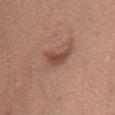This lesion was catalogued during total-body skin photography and was not selected for biopsy.
On the upper back.
The recorded lesion diameter is about 3 mm.
The tile uses white-light illumination.
The lesion-visualizer software estimated an automated nevus-likeness rating near 80 out of 100 and a lesion-detection confidence of about 100/100.
The subject is a female roughly 65 years of age.
Cropped from a total-body skin-imaging series; the visible field is about 15 mm.